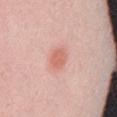notes = total-body-photography surveillance lesion; no biopsy | location = the abdomen | image = ~15 mm crop, total-body skin-cancer survey | subject = female, approximately 15 years of age.